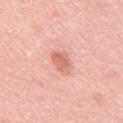  biopsy_status: not biopsied; imaged during a skin examination
  image:
    source: total-body photography crop
    field_of_view_mm: 15
  patient:
    sex: male
    age_approx: 50
  site: back
  lighting: white-light